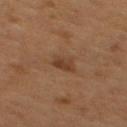This lesion was catalogued during total-body skin photography and was not selected for biopsy. Measured at roughly 2.5 mm in maximum diameter. Automated tile analysis of the lesion measured an average lesion color of about L≈35 a*≈18 b*≈28 (CIELAB). A male patient in their mid-50s. The lesion is located on the mid back. Captured under cross-polarized illumination. A close-up tile cropped from a whole-body skin photograph, about 15 mm across.